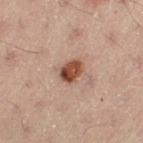This lesion was catalogued during total-body skin photography and was not selected for biopsy. On the left thigh. A close-up tile cropped from a whole-body skin photograph, about 15 mm across. The subject is a male in their 50s.